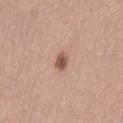Imaged during a routine full-body skin examination; the lesion was not biopsied and no histopathology is available. A 15 mm close-up extracted from a 3D total-body photography capture. A female patient, in their 40s. The lesion is located on the leg.